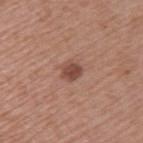Case summary:
– biopsy status — catalogued during a skin exam; not biopsied
– automated metrics — about 11 CIELAB-L* units darker than the surrounding skin and a normalized lesion–skin contrast near 8.5; border irregularity of about 1.5 on a 0–10 scale, a color-variation rating of about 2.5/10, and a peripheral color-asymmetry measure near 1
– subject — female, in their mid- to late 40s
– acquisition — total-body-photography crop, ~15 mm field of view
– diameter — ~2.5 mm (longest diameter)
– site — the left upper arm